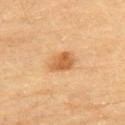Imaged during a routine full-body skin examination; the lesion was not biopsied and no histopathology is available.
On the back.
Longest diameter approximately 3.5 mm.
A male patient in their mid- to late 80s or older.
This is a cross-polarized tile.
Cropped from a whole-body photographic skin survey; the tile spans about 15 mm.